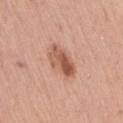The recorded lesion diameter is about 4.5 mm. A 15 mm crop from a total-body photograph taken for skin-cancer surveillance. From the lower back. A female subject approximately 55 years of age.